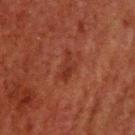Impression:
Captured during whole-body skin photography for melanoma surveillance; the lesion was not biopsied.
Acquisition and patient details:
Located on the upper back. A male subject, aged 58 to 62. Longest diameter approximately 3.5 mm. A roughly 15 mm field-of-view crop from a total-body skin photograph.